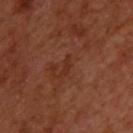| feature | finding |
|---|---|
| workup | total-body-photography surveillance lesion; no biopsy |
| patient | male, in their 50s |
| lesion size | ≈2.5 mm |
| image source | ~15 mm crop, total-body skin-cancer survey |
| location | the upper back |